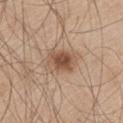This lesion was catalogued during total-body skin photography and was not selected for biopsy.
Imaged with white-light lighting.
The lesion is on the leg.
A male patient about 60 years old.
The lesion-visualizer software estimated an area of roughly 12 mm², an outline eccentricity of about 0.75 (0 = round, 1 = elongated), and a symmetry-axis asymmetry near 0.2. The software also gave a lesion–skin lightness drop of about 11 and a normalized lesion–skin contrast near 7.5. The analysis additionally found a nevus-likeness score of about 95/100 and a detector confidence of about 100 out of 100 that the crop contains a lesion.
A lesion tile, about 15 mm wide, cut from a 3D total-body photograph.